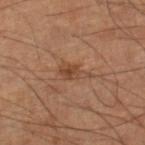notes: no biopsy performed (imaged during a skin exam)
imaging modality: ~15 mm tile from a whole-body skin photo
lighting: cross-polarized
TBP lesion metrics: a lesion color around L≈43 a*≈20 b*≈30 in CIELAB, a lesion–skin lightness drop of about 8, and a normalized border contrast of about 6.5; a nevus-likeness score of about 30/100 and a lesion-detection confidence of about 100/100
subject: male, approximately 60 years of age
anatomic site: the right lower leg